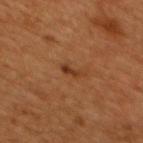workup: total-body-photography surveillance lesion; no biopsy | automated lesion analysis: a detector confidence of about 100 out of 100 that the crop contains a lesion | imaging modality: ~15 mm tile from a whole-body skin photo | lighting: cross-polarized illumination | subject: male, aged approximately 45 | body site: the mid back | lesion diameter: ≈2.5 mm.